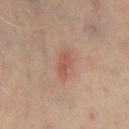  biopsy_status: not biopsied; imaged during a skin examination
  site: chest
  lesion_size:
    long_diameter_mm_approx: 3.0
  patient:
    sex: male
    age_approx: 60
  lighting: cross-polarized
  image:
    source: total-body photography crop
    field_of_view_mm: 15